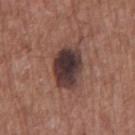The lesion was photographed on a routine skin check and not biopsied; there is no pathology result.
A male subject approximately 75 years of age.
The lesion-visualizer software estimated a lesion area of about 13 mm² and a shape-asymmetry score of about 0.15 (0 = symmetric). And it measured border irregularity of about 1.5 on a 0–10 scale and a peripheral color-asymmetry measure near 1.5. The analysis additionally found a classifier nevus-likeness of about 20/100 and a detector confidence of about 100 out of 100 that the crop contains a lesion.
A lesion tile, about 15 mm wide, cut from a 3D total-body photograph.
On the chest.
About 4.5 mm across.